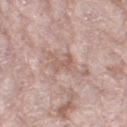Q: How large is the lesion?
A: about 2.5 mm
Q: Illumination type?
A: white-light
Q: Who is the patient?
A: female, aged 68 to 72
Q: How was this image acquired?
A: 15 mm crop, total-body photography
Q: What is the anatomic site?
A: the right thigh
Q: Automated lesion metrics?
A: an eccentricity of roughly 0.75; a mean CIELAB color near L≈58 a*≈18 b*≈25, roughly 7 lightness units darker than nearby skin, and a normalized border contrast of about 5.5; a border-irregularity rating of about 5.5/10, a color-variation rating of about 0/10, and peripheral color asymmetry of about 0; a classifier nevus-likeness of about 0/100 and a detector confidence of about 70 out of 100 that the crop contains a lesion